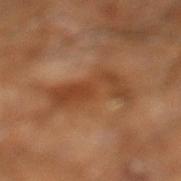follow-up: catalogued during a skin exam; not biopsied
location: the right leg
diameter: ~6.5 mm (longest diameter)
lighting: cross-polarized
imaging modality: ~15 mm crop, total-body skin-cancer survey
automated lesion analysis: a footprint of about 14 mm² and an eccentricity of roughly 0.9; about 8 CIELAB-L* units darker than the surrounding skin and a lesion-to-skin contrast of about 7 (normalized; higher = more distinct); a border-irregularity index near 7/10 and internal color variation of about 3 on a 0–10 scale
subject: male, aged around 60